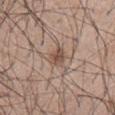Impression: Part of a total-body skin-imaging series; this lesion was reviewed on a skin check and was not flagged for biopsy. Clinical summary: From the mid back. Approximately 2.5 mm at its widest. A male subject approximately 45 years of age. This image is a 15 mm lesion crop taken from a total-body photograph.